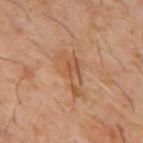Recorded during total-body skin imaging; not selected for excision or biopsy.
Imaged with cross-polarized lighting.
A region of skin cropped from a whole-body photographic capture, roughly 15 mm wide.
Automated image analysis of the tile measured an area of roughly 11 mm², an eccentricity of roughly 0.9, and a shape-asymmetry score of about 0.4 (0 = symmetric). The analysis additionally found a lesion color around L≈50 a*≈20 b*≈32 in CIELAB, a lesion–skin lightness drop of about 7, and a lesion-to-skin contrast of about 5.5 (normalized; higher = more distinct).
A male patient aged approximately 60.
On the mid back.